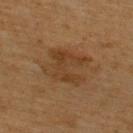notes: total-body-photography surveillance lesion; no biopsy
illumination: cross-polarized
image: ~15 mm crop, total-body skin-cancer survey
subject: male, aged around 65
body site: the upper back